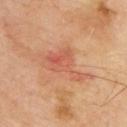Assessment: Imaged during a routine full-body skin examination; the lesion was not biopsied and no histopathology is available. Context: Longest diameter approximately 6.5 mm. A roughly 15 mm field-of-view crop from a total-body skin photograph. A male subject, in their mid-60s. Located on the upper back. This is a cross-polarized tile.